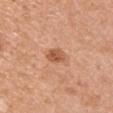This lesion was catalogued during total-body skin photography and was not selected for biopsy.
The lesion is on the left upper arm.
A female subject in their 50s.
Cropped from a whole-body photographic skin survey; the tile spans about 15 mm.